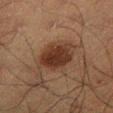Imaged during a routine full-body skin examination; the lesion was not biopsied and no histopathology is available.
This is a cross-polarized tile.
A 15 mm close-up extracted from a 3D total-body photography capture.
Automated image analysis of the tile measured about 10 CIELAB-L* units darker than the surrounding skin and a lesion-to-skin contrast of about 10.5 (normalized; higher = more distinct). It also reported a border-irregularity index near 2/10, a color-variation rating of about 3/10, and a peripheral color-asymmetry measure near 1. And it measured a nevus-likeness score of about 90/100 and a lesion-detection confidence of about 100/100.
On the leg.
Approximately 4 mm at its widest.
A male subject about 50 years old.